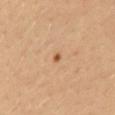{"biopsy_status": "not biopsied; imaged during a skin examination", "site": "mid back", "patient": {"sex": "male", "age_approx": 35}, "lesion_size": {"long_diameter_mm_approx": 1.0}, "image": {"source": "total-body photography crop", "field_of_view_mm": 15}, "lighting": "cross-polarized", "automated_metrics": {"color_variation_0_10": 0.0, "peripheral_color_asymmetry": 0.0, "nevus_likeness_0_100": 55, "lesion_detection_confidence_0_100": 100}}